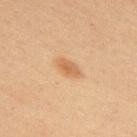The tile uses cross-polarized illumination.
On the upper back.
Longest diameter approximately 3 mm.
A lesion tile, about 15 mm wide, cut from a 3D total-body photograph.
The patient is a male roughly 60 years of age.